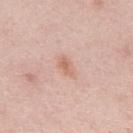Assessment:
No biopsy was performed on this lesion — it was imaged during a full skin examination and was not determined to be concerning.
Background:
A male subject, roughly 25 years of age. From the upper back. Automated tile analysis of the lesion measured a footprint of about 2.5 mm², an outline eccentricity of about 0.85 (0 = round, 1 = elongated), and a shape-asymmetry score of about 0.3 (0 = symmetric). The software also gave a lesion color around L≈64 a*≈22 b*≈30 in CIELAB and a normalized border contrast of about 6.5. And it measured a border-irregularity index near 3/10, a color-variation rating of about 0.5/10, and a peripheral color-asymmetry measure near 0. Longest diameter approximately 2.5 mm. The tile uses white-light illumination. A 15 mm crop from a total-body photograph taken for skin-cancer surveillance.